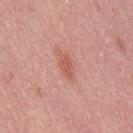<case>
  <biopsy_status>not biopsied; imaged during a skin examination</biopsy_status>
  <patient>
    <sex>male</sex>
    <age_approx>40</age_approx>
  </patient>
  <image>
    <source>total-body photography crop</source>
    <field_of_view_mm>15</field_of_view_mm>
  </image>
  <site>right thigh</site>
  <lesion_size>
    <long_diameter_mm_approx>3.0</long_diameter_mm_approx>
  </lesion_size>
  <automated_metrics>
    <eccentricity>0.85</eccentricity>
    <shape_asymmetry>0.2</shape_asymmetry>
    <nevus_likeness_0_100>65</nevus_likeness_0_100>
    <lesion_detection_confidence_0_100>100</lesion_detection_confidence_0_100>
  </automated_metrics>
  <lighting>white-light</lighting>
</case>